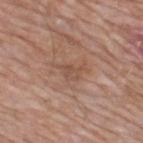workup = catalogued during a skin exam; not biopsied | image = ~15 mm tile from a whole-body skin photo | diameter = ≈3.5 mm | automated metrics = a lesion–skin lightness drop of about 7 and a normalized lesion–skin contrast near 5; an automated nevus-likeness rating near 0 out of 100 | anatomic site = the upper back | illumination = white-light illumination | patient = male, roughly 60 years of age.